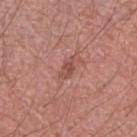| feature | finding |
|---|---|
| workup | total-body-photography surveillance lesion; no biopsy |
| body site | the arm |
| image source | ~15 mm tile from a whole-body skin photo |
| subject | male, approximately 40 years of age |